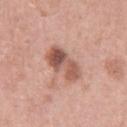Part of a total-body skin-imaging series; this lesion was reviewed on a skin check and was not flagged for biopsy.
The lesion is located on the right thigh.
A female patient, about 55 years old.
A roughly 15 mm field-of-view crop from a total-body skin photograph.
Captured under white-light illumination.
The recorded lesion diameter is about 4.5 mm.